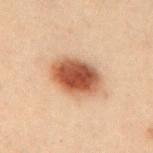Assessment:
Part of a total-body skin-imaging series; this lesion was reviewed on a skin check and was not flagged for biopsy.
Context:
Approximately 5.5 mm at its widest. The lesion is located on the mid back. The subject is a female roughly 30 years of age. Automated tile analysis of the lesion measured border irregularity of about 1.5 on a 0–10 scale, a color-variation rating of about 6.5/10, and radial color variation of about 1.5. And it measured a nevus-likeness score of about 100/100 and lesion-presence confidence of about 100/100. A roughly 15 mm field-of-view crop from a total-body skin photograph.